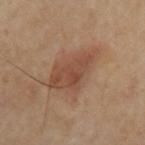Notes:
* notes — no biopsy performed (imaged during a skin exam)
* size — ~6.5 mm (longest diameter)
* location — the left upper arm
* image source — ~15 mm tile from a whole-body skin photo
* tile lighting — cross-polarized
* automated metrics — a lesion color around L≈49 a*≈20 b*≈30 in CIELAB, a lesion–skin lightness drop of about 9, and a normalized border contrast of about 6.5